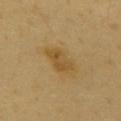A region of skin cropped from a whole-body photographic capture, roughly 15 mm wide. The tile uses cross-polarized illumination. A male subject aged 63–67. Automated tile analysis of the lesion measured a normalized lesion–skin contrast near 6.5. It also reported a border-irregularity rating of about 3.5/10, a color-variation rating of about 3/10, and peripheral color asymmetry of about 1. On the mid back.